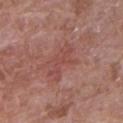Captured during whole-body skin photography for melanoma surveillance; the lesion was not biopsied. The lesion is located on the arm. The recorded lesion diameter is about 4.5 mm. The tile uses white-light illumination. The subject is a female aged approximately 70. An algorithmic analysis of the crop reported a lesion color around L≈48 a*≈25 b*≈24 in CIELAB, about 6 CIELAB-L* units darker than the surrounding skin, and a lesion-to-skin contrast of about 5 (normalized; higher = more distinct). And it measured a color-variation rating of about 1/10 and a peripheral color-asymmetry measure near 0.5. It also reported a nevus-likeness score of about 0/100. A 15 mm crop from a total-body photograph taken for skin-cancer surveillance.